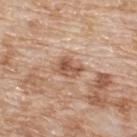Recorded during total-body skin imaging; not selected for excision or biopsy. Captured under white-light illumination. A 15 mm close-up tile from a total-body photography series done for melanoma screening. The lesion is on the upper back. An algorithmic analysis of the crop reported an area of roughly 5 mm² and a symmetry-axis asymmetry near 0.3. It also reported a mean CIELAB color near L≈55 a*≈21 b*≈31. It also reported a border-irregularity rating of about 3/10 and a within-lesion color-variation index near 5/10. A male patient approximately 80 years of age.